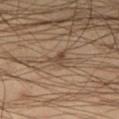{"biopsy_status": "not biopsied; imaged during a skin examination", "image": {"source": "total-body photography crop", "field_of_view_mm": 15}, "lesion_size": {"long_diameter_mm_approx": 2.5}, "lighting": "cross-polarized", "site": "right thigh", "patient": {"sex": "male", "age_approx": 45}}